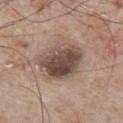Notes:
– biopsy status · no biopsy performed (imaged during a skin exam)
– lesion size · ~5.5 mm (longest diameter)
– lighting · white-light illumination
– automated metrics · an area of roughly 20 mm² and a symmetry-axis asymmetry near 0.2; roughly 14 lightness units darker than nearby skin and a normalized border contrast of about 10.5
– patient · male, aged 63 to 67
– location · the arm
– imaging modality · ~15 mm tile from a whole-body skin photo